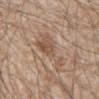Background:
A close-up tile cropped from a whole-body skin photograph, about 15 mm across. The lesion-visualizer software estimated an area of roughly 11 mm², an outline eccentricity of about 0.95 (0 = round, 1 = elongated), and a symmetry-axis asymmetry near 0.5. And it measured an automated nevus-likeness rating near 0 out of 100 and lesion-presence confidence of about 70/100. Measured at roughly 7 mm in maximum diameter. A male patient, aged approximately 80. Captured under white-light illumination. The lesion is located on the abdomen.